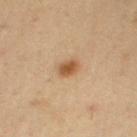<tbp_lesion>
<biopsy_status>not biopsied; imaged during a skin examination</biopsy_status>
<site>left upper arm</site>
<patient>
  <sex>male</sex>
  <age_approx>55</age_approx>
</patient>
<image>
  <source>total-body photography crop</source>
  <field_of_view_mm>15</field_of_view_mm>
</image>
</tbp_lesion>